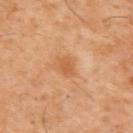Case summary:
– diameter: ≈2.5 mm
– anatomic site: the upper back
– image: total-body-photography crop, ~15 mm field of view
– patient: male, about 60 years old
– illumination: cross-polarized
– automated lesion analysis: a mean CIELAB color near L≈53 a*≈24 b*≈38; a border-irregularity rating of about 2.5/10 and peripheral color asymmetry of about 1; an automated nevus-likeness rating near 10 out of 100